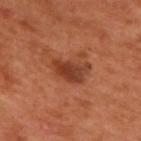notes: catalogued during a skin exam; not biopsied | patient: male, aged around 50 | location: the upper back | illumination: cross-polarized | automated lesion analysis: an area of roughly 8.5 mm², an eccentricity of roughly 0.8, and a symmetry-axis asymmetry near 0.45; about 11 CIELAB-L* units darker than the surrounding skin and a normalized lesion–skin contrast near 8.5; a classifier nevus-likeness of about 55/100 and a lesion-detection confidence of about 100/100 | imaging modality: ~15 mm tile from a whole-body skin photo | size: ≈4.5 mm.